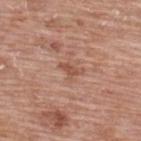| feature | finding |
|---|---|
| workup | imaged on a skin check; not biopsied |
| image | ~15 mm crop, total-body skin-cancer survey |
| subject | female, in their 60s |
| anatomic site | the upper back |